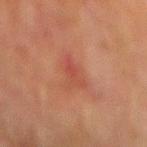Background: Captured under cross-polarized illumination. The patient is a male approximately 75 years of age. A 15 mm close-up tile from a total-body photography series done for melanoma screening. Longest diameter approximately 3 mm. The lesion is on the mid back.